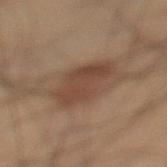{"biopsy_status": "not biopsied; imaged during a skin examination", "lighting": "cross-polarized", "automated_metrics": {"eccentricity": 0.25, "shape_asymmetry": 0.15, "vs_skin_contrast_norm": 7.0, "color_variation_0_10": 2.5, "peripheral_color_asymmetry": 1.0, "lesion_detection_confidence_0_100": 100}, "site": "left lower leg", "image": {"source": "total-body photography crop", "field_of_view_mm": 15}, "patient": {"sex": "male", "age_approx": 55}}